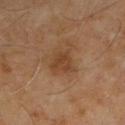{
  "biopsy_status": "not biopsied; imaged during a skin examination",
  "patient": {
    "sex": "male",
    "age_approx": 55
  },
  "site": "back",
  "lighting": "cross-polarized",
  "lesion_size": {
    "long_diameter_mm_approx": 3.0
  },
  "image": {
    "source": "total-body photography crop",
    "field_of_view_mm": 15
  }
}